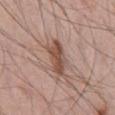biopsy_status: not biopsied; imaged during a skin examination
site: mid back
image:
  source: total-body photography crop
  field_of_view_mm: 15
patient:
  sex: male
  age_approx: 45
lighting: white-light
lesion_size:
  long_diameter_mm_approx: 4.5